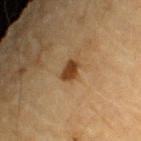Captured during whole-body skin photography for melanoma surveillance; the lesion was not biopsied.
Cropped from a total-body skin-imaging series; the visible field is about 15 mm.
A male subject in their mid- to late 80s or older.
Approximately 2.5 mm at its widest.
Located on the arm.
Imaged with cross-polarized lighting.